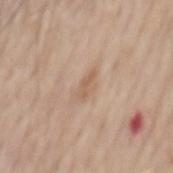No biopsy was performed on this lesion — it was imaged during a full skin examination and was not determined to be concerning.
A male subject aged around 65.
The lesion's longest dimension is about 3 mm.
The lesion is on the back.
A 15 mm crop from a total-body photograph taken for skin-cancer surveillance.
Imaged with white-light lighting.
Automated image analysis of the tile measured a footprint of about 4 mm². The software also gave a lesion–skin lightness drop of about 7 and a normalized lesion–skin contrast near 5. It also reported a classifier nevus-likeness of about 0/100 and a lesion-detection confidence of about 100/100.